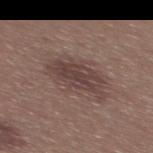notes: catalogued during a skin exam; not biopsied | subject: female, aged 23–27 | image source: ~15 mm tile from a whole-body skin photo | location: the upper back.